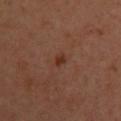Impression: Recorded during total-body skin imaging; not selected for excision or biopsy. Clinical summary: The lesion is located on the back. A 15 mm close-up tile from a total-body photography series done for melanoma screening. The lesion's longest dimension is about 2 mm. The total-body-photography lesion software estimated an eccentricity of roughly 0.7 and a shape-asymmetry score of about 0.35 (0 = symmetric). The analysis additionally found an average lesion color of about L≈32 a*≈23 b*≈27 (CIELAB), a lesion–skin lightness drop of about 7, and a normalized lesion–skin contrast near 7.5. And it measured a peripheral color-asymmetry measure near 0. A female patient, aged approximately 40.